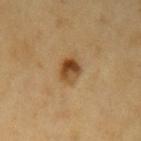• lighting: cross-polarized
• body site: the left upper arm
• acquisition: ~15 mm tile from a whole-body skin photo
• size: ≈3 mm
• patient: male, approximately 60 years of age